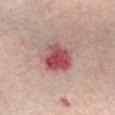biopsy status — catalogued during a skin exam; not biopsied | anatomic site — the chest | patient — female, approximately 65 years of age | lesion diameter — ~4 mm (longest diameter) | illumination — white-light illumination | automated lesion analysis — a footprint of about 11 mm², an outline eccentricity of about 0.4 (0 = round, 1 = elongated), and a shape-asymmetry score of about 0.2 (0 = symmetric); an average lesion color of about L≈52 a*≈31 b*≈20 (CIELAB), a lesion–skin lightness drop of about 15, and a normalized border contrast of about 10; a color-variation rating of about 7.5/10 and radial color variation of about 2.5 | image — total-body-photography crop, ~15 mm field of view.